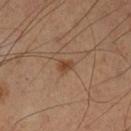anatomic site: the left lower leg
patient: male, roughly 55 years of age
lesion size: ~2 mm (longest diameter)
illumination: cross-polarized illumination
TBP lesion metrics: a lesion area of about 2.5 mm², an eccentricity of roughly 0.7, and a shape-asymmetry score of about 0.25 (0 = symmetric); a lesion color around L≈43 a*≈20 b*≈31 in CIELAB, about 9 CIELAB-L* units darker than the surrounding skin, and a lesion-to-skin contrast of about 7.5 (normalized; higher = more distinct); a border-irregularity index near 2/10; a classifier nevus-likeness of about 90/100 and a lesion-detection confidence of about 100/100
acquisition: ~15 mm tile from a whole-body skin photo